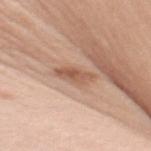Imaged during a routine full-body skin examination; the lesion was not biopsied and no histopathology is available.
From the right upper arm.
This is a white-light tile.
The patient is a female approximately 50 years of age.
This image is a 15 mm lesion crop taken from a total-body photograph.